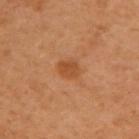notes — no biopsy performed (imaged during a skin exam)
acquisition — 15 mm crop, total-body photography
site — the right arm
subject — female, roughly 60 years of age
illumination — cross-polarized illumination
automated lesion analysis — border irregularity of about 1.5 on a 0–10 scale and a within-lesion color-variation index near 2/10